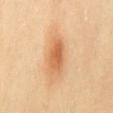The lesion was photographed on a routine skin check and not biopsied; there is no pathology result. Located on the mid back. A female patient aged 38–42. The total-body-photography lesion software estimated an eccentricity of roughly 0.85 and a shape-asymmetry score of about 0.1 (0 = symmetric). The software also gave a lesion color around L≈64 a*≈24 b*≈41 in CIELAB and roughly 11 lightness units darker than nearby skin. Imaged with cross-polarized lighting. About 5 mm across. Cropped from a whole-body photographic skin survey; the tile spans about 15 mm.